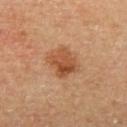Clinical impression:
This lesion was catalogued during total-body skin photography and was not selected for biopsy.
Clinical summary:
This is a cross-polarized tile. The total-body-photography lesion software estimated a symmetry-axis asymmetry near 0.2. The analysis additionally found a border-irregularity index near 2.5/10, a color-variation rating of about 6/10, and a peripheral color-asymmetry measure near 2.5. The software also gave a lesion-detection confidence of about 100/100. Cropped from a total-body skin-imaging series; the visible field is about 15 mm. The subject is a male in their mid-60s. The lesion is on the left upper arm.